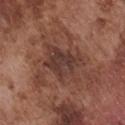Recorded during total-body skin imaging; not selected for excision or biopsy. Located on the chest. The subject is a male in their mid-70s. The lesion-visualizer software estimated an area of roughly 13 mm², an outline eccentricity of about 0.6 (0 = round, 1 = elongated), and a shape-asymmetry score of about 0.35 (0 = symmetric). Captured under white-light illumination. Approximately 5 mm at its widest. A region of skin cropped from a whole-body photographic capture, roughly 15 mm wide.